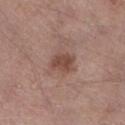workup: total-body-photography surveillance lesion; no biopsy | subject: male, in their mid-50s | lighting: white-light | diameter: ~3 mm (longest diameter) | location: the right lower leg | image-analysis metrics: a lesion area of about 6 mm², an outline eccentricity of about 0.7 (0 = round, 1 = elongated), and a symmetry-axis asymmetry near 0.15; a lesion color around L≈46 a*≈21 b*≈25 in CIELAB and roughly 10 lightness units darker than nearby skin; border irregularity of about 1.5 on a 0–10 scale and peripheral color asymmetry of about 0.5 | image source: ~15 mm tile from a whole-body skin photo.